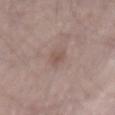notes = total-body-photography surveillance lesion; no biopsy | patient = male, aged around 65 | body site = the mid back | image = ~15 mm crop, total-body skin-cancer survey.